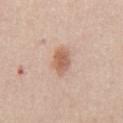{
  "biopsy_status": "not biopsied; imaged during a skin examination",
  "site": "abdomen",
  "image": {
    "source": "total-body photography crop",
    "field_of_view_mm": 15
  },
  "patient": {
    "sex": "male",
    "age_approx": 60
  }
}